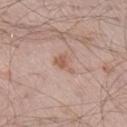notes: catalogued during a skin exam; not biopsied
lighting: white-light illumination
image: 15 mm crop, total-body photography
TBP lesion metrics: a shape eccentricity near 0.75 and two-axis asymmetry of about 0.6; a mean CIELAB color near L≈58 a*≈20 b*≈27, about 9 CIELAB-L* units darker than the surrounding skin, and a normalized border contrast of about 6.5
patient: male, about 60 years old
lesion diameter: ≈3 mm
anatomic site: the right lower leg